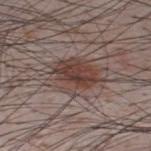Captured during whole-body skin photography for melanoma surveillance; the lesion was not biopsied.
A close-up tile cropped from a whole-body skin photograph, about 15 mm across.
The subject is a male roughly 35 years of age.
Imaged with white-light lighting.
The lesion-visualizer software estimated a lesion area of about 15 mm², an eccentricity of roughly 0.7, and two-axis asymmetry of about 0.2. The software also gave an average lesion color of about L≈41 a*≈17 b*≈21 (CIELAB), about 10 CIELAB-L* units darker than the surrounding skin, and a normalized border contrast of about 8.5.
About 5.5 mm across.
On the upper back.